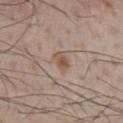Impression:
The lesion was tiled from a total-body skin photograph and was not biopsied.
Context:
The patient is a male aged approximately 50. A region of skin cropped from a whole-body photographic capture, roughly 15 mm wide. Approximately 2.5 mm at its widest. Captured under white-light illumination. Automated tile analysis of the lesion measured a lesion area of about 4 mm², an outline eccentricity of about 0.75 (0 = round, 1 = elongated), and two-axis asymmetry of about 0.25. And it measured a border-irregularity index near 2.5/10 and peripheral color asymmetry of about 1. The analysis additionally found an automated nevus-likeness rating near 75 out of 100 and a detector confidence of about 100 out of 100 that the crop contains a lesion. From the chest.